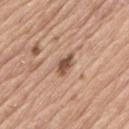No biopsy was performed on this lesion — it was imaged during a full skin examination and was not determined to be concerning. Cropped from a whole-body photographic skin survey; the tile spans about 15 mm. A male subject, roughly 70 years of age. On the left thigh.